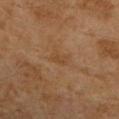biopsy_status: not biopsied; imaged during a skin examination
lighting: cross-polarized
site: right upper arm
patient:
  sex: female
  age_approx: 60
automated_metrics:
  area_mm2_approx: 3.0
  eccentricity: 0.85
  shape_asymmetry: 0.3
  cielab_L: 41
  cielab_a: 18
  cielab_b: 32
  vs_skin_darker_L: 5.0
  border_irregularity_0_10: 3.0
  color_variation_0_10: 1.0
  peripheral_color_asymmetry: 0.0
  nevus_likeness_0_100: 0
image:
  source: total-body photography crop
  field_of_view_mm: 15
lesion_size:
  long_diameter_mm_approx: 2.5